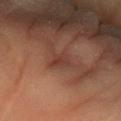<case>
  <biopsy_status>not biopsied; imaged during a skin examination</biopsy_status>
  <patient>
    <sex>male</sex>
    <age_approx>65</age_approx>
  </patient>
  <site>left forearm</site>
  <image>
    <source>total-body photography crop</source>
    <field_of_view_mm>15</field_of_view_mm>
  </image>
</case>